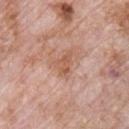Clinical impression: Imaged during a routine full-body skin examination; the lesion was not biopsied and no histopathology is available. Image and clinical context: A roughly 15 mm field-of-view crop from a total-body skin photograph. Imaged with white-light lighting. A male subject, aged around 70. From the front of the torso. Automated image analysis of the tile measured an area of roughly 5 mm² and a shape eccentricity near 0.7. The analysis additionally found a mean CIELAB color near L≈57 a*≈23 b*≈31, roughly 8 lightness units darker than nearby skin, and a lesion-to-skin contrast of about 6 (normalized; higher = more distinct). The analysis additionally found a border-irregularity rating of about 5/10, a color-variation rating of about 3.5/10, and a peripheral color-asymmetry measure near 1. The analysis additionally found lesion-presence confidence of about 100/100.